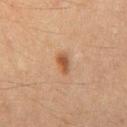workup: catalogued during a skin exam; not biopsied
patient: male, aged around 60
acquisition: ~15 mm tile from a whole-body skin photo
site: the chest